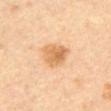biopsy_status: not biopsied; imaged during a skin examination
automated_metrics:
  area_mm2_approx: 8.5
  eccentricity: 0.6
  border_irregularity_0_10: 2.5
  color_variation_0_10: 5.0
  nevus_likeness_0_100: 55
  lesion_detection_confidence_0_100: 100
patient:
  sex: female
  age_approx: 55
image:
  source: total-body photography crop
  field_of_view_mm: 15
lighting: cross-polarized
lesion_size:
  long_diameter_mm_approx: 4.0
site: abdomen